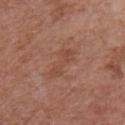Impression: No biopsy was performed on this lesion — it was imaged during a full skin examination and was not determined to be concerning. Background: From the chest. Cropped from a whole-body photographic skin survey; the tile spans about 15 mm. Approximately 4 mm at its widest. The tile uses white-light illumination. A male subject in their mid-50s.